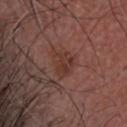workup = catalogued during a skin exam; not biopsied | subject = male, aged around 65 | tile lighting = cross-polarized | lesion size = ~3.5 mm (longest diameter) | location = the head or neck | image = ~15 mm tile from a whole-body skin photo.